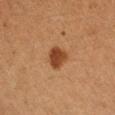image: ~15 mm crop, total-body skin-cancer survey | site: the left upper arm | patient: female, aged around 40.